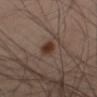workup: imaged on a skin check; not biopsied
tile lighting: cross-polarized illumination
subject: male, aged around 60
size: ≈3 mm
TBP lesion metrics: an eccentricity of roughly 0.8 and a shape-asymmetry score of about 0.3 (0 = symmetric); a mean CIELAB color near L≈32 a*≈17 b*≈24, a lesion–skin lightness drop of about 10, and a normalized lesion–skin contrast near 10.5; a classifier nevus-likeness of about 100/100 and a lesion-detection confidence of about 100/100
site: the right thigh
acquisition: ~15 mm crop, total-body skin-cancer survey